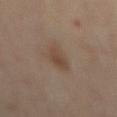Clinical impression: The lesion was tiled from a total-body skin photograph and was not biopsied. Clinical summary: This image is a 15 mm lesion crop taken from a total-body photograph. From the back. Imaged with cross-polarized lighting. The recorded lesion diameter is about 3.5 mm. An algorithmic analysis of the crop reported a border-irregularity rating of about 2.5/10, internal color variation of about 2.5 on a 0–10 scale, and radial color variation of about 1. It also reported an automated nevus-likeness rating near 80 out of 100 and a lesion-detection confidence of about 100/100. A female subject aged 58 to 62.